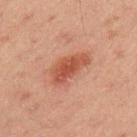Part of a total-body skin-imaging series; this lesion was reviewed on a skin check and was not flagged for biopsy. The lesion is on the left upper arm. A male patient about 45 years old. A lesion tile, about 15 mm wide, cut from a 3D total-body photograph.